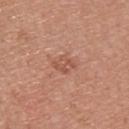Notes:
• follow-up — catalogued during a skin exam; not biopsied
• diameter — about 2.5 mm
• tile lighting — white-light
• site — the upper back
• image source — 15 mm crop, total-body photography
• patient — male, roughly 40 years of age